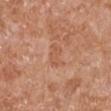Clinical impression: The lesion was photographed on a routine skin check and not biopsied; there is no pathology result. Clinical summary: Captured under white-light illumination. Located on the right upper arm. Cropped from a whole-body photographic skin survey; the tile spans about 15 mm. A male patient, aged 63 to 67.